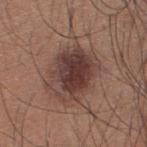biopsy_status: not biopsied; imaged during a skin examination
lighting: white-light
patient:
  sex: male
  age_approx: 35
lesion_size:
  long_diameter_mm_approx: 6.0
image:
  source: total-body photography crop
  field_of_view_mm: 15
automated_metrics:
  area_mm2_approx: 21.0
  eccentricity: 0.65
  border_irregularity_0_10: 2.5
  color_variation_0_10: 6.5
  peripheral_color_asymmetry: 2.0
site: back